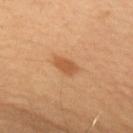From the right upper arm. A male patient aged around 40. A roughly 15 mm field-of-view crop from a total-body skin photograph.